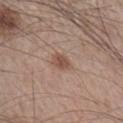This lesion was catalogued during total-body skin photography and was not selected for biopsy. A 15 mm close-up extracted from a 3D total-body photography capture. Imaged with white-light lighting. The recorded lesion diameter is about 2.5 mm. A male subject, aged around 45. The lesion is on the right lower leg.